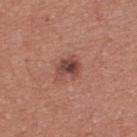| field | value |
|---|---|
| follow-up | imaged on a skin check; not biopsied |
| lesion size | about 3.5 mm |
| automated lesion analysis | an area of roughly 6.5 mm² and an outline eccentricity of about 0.7 (0 = round, 1 = elongated); a lesion color around L≈45 a*≈23 b*≈25 in CIELAB, about 11 CIELAB-L* units darker than the surrounding skin, and a normalized lesion–skin contrast near 8.5; border irregularity of about 3.5 on a 0–10 scale, a color-variation rating of about 6.5/10, and radial color variation of about 2; a nevus-likeness score of about 50/100 |
| lighting | white-light |
| anatomic site | the upper back |
| imaging modality | ~15 mm tile from a whole-body skin photo |
| subject | male, aged around 40 |